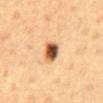biopsy_status: not biopsied; imaged during a skin examination
site: abdomen
image:
  source: total-body photography crop
  field_of_view_mm: 15
automated_metrics:
  area_mm2_approx: 6.0
  shape_asymmetry: 0.25
  vs_skin_darker_L: 20.0
  vs_skin_contrast_norm: 13.0
  border_irregularity_0_10: 2.0
  color_variation_0_10: 9.0
  peripheral_color_asymmetry: 2.5
  nevus_likeness_0_100: 100
  lesion_detection_confidence_0_100: 100
lesion_size:
  long_diameter_mm_approx: 3.0
patient:
  sex: male
  age_approx: 60